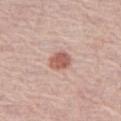• location · the right thigh
• image source · ~15 mm tile from a whole-body skin photo
• illumination · white-light illumination
• patient · female, about 70 years old
• image-analysis metrics · an eccentricity of roughly 0.6; a lesion color around L≈57 a*≈24 b*≈26 in CIELAB, roughly 13 lightness units darker than nearby skin, and a normalized lesion–skin contrast near 8.5; a classifier nevus-likeness of about 95/100